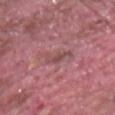Part of a total-body skin-imaging series; this lesion was reviewed on a skin check and was not flagged for biopsy.
A male patient, roughly 40 years of age.
The lesion's longest dimension is about 3 mm.
The total-body-photography lesion software estimated about 8 CIELAB-L* units darker than the surrounding skin and a normalized lesion–skin contrast near 5.5. And it measured a border-irregularity index near 4.5/10, a within-lesion color-variation index near 1/10, and radial color variation of about 0.5. The analysis additionally found a nevus-likeness score of about 0/100.
The lesion is on the arm.
This image is a 15 mm lesion crop taken from a total-body photograph.